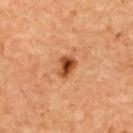| key | value |
|---|---|
| follow-up | no biopsy performed (imaged during a skin exam) |
| anatomic site | the back |
| acquisition | 15 mm crop, total-body photography |
| tile lighting | cross-polarized illumination |
| subject | female, about 60 years old |
| diameter | ≈3 mm |